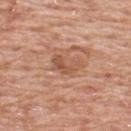No biopsy was performed on this lesion — it was imaged during a full skin examination and was not determined to be concerning.
The lesion-visualizer software estimated an outline eccentricity of about 0.8 (0 = round, 1 = elongated) and a shape-asymmetry score of about 0.4 (0 = symmetric). The software also gave a border-irregularity index near 4.5/10 and a within-lesion color-variation index near 4/10. The analysis additionally found a lesion-detection confidence of about 100/100.
A region of skin cropped from a whole-body photographic capture, roughly 15 mm wide.
The lesion is on the upper back.
The subject is a male aged 58 to 62.